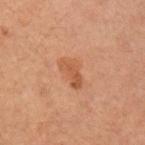This lesion was catalogued during total-body skin photography and was not selected for biopsy. From the right upper arm. A 15 mm crop from a total-body photograph taken for skin-cancer surveillance. A male subject in their 60s. Measured at roughly 4 mm in maximum diameter. The total-body-photography lesion software estimated a classifier nevus-likeness of about 5/100.